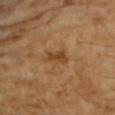biopsy_status: not biopsied; imaged during a skin examination
automated_metrics:
  vs_skin_contrast_norm: 7.5
  border_irregularity_0_10: 3.5
  color_variation_0_10: 1.5
  peripheral_color_asymmetry: 0.5
  lesion_detection_confidence_0_100: 100
patient:
  sex: female
  age_approx: 70
lighting: cross-polarized
image:
  source: total-body photography crop
  field_of_view_mm: 15
lesion_size:
  long_diameter_mm_approx: 3.0
site: right upper arm